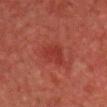lighting=cross-polarized illumination | image source=~15 mm tile from a whole-body skin photo | site=the head or neck | subject=male, roughly 45 years of age.